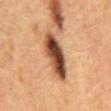workup = imaged on a skin check; not biopsied | patient = male, aged around 65 | image = ~15 mm crop, total-body skin-cancer survey | diameter = ≈6.5 mm | illumination = cross-polarized illumination | image-analysis metrics = a lesion color around L≈39 a*≈20 b*≈28 in CIELAB, roughly 19 lightness units darker than nearby skin, and a normalized border contrast of about 14 | site = the mid back.